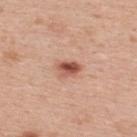Captured during whole-body skin photography for melanoma surveillance; the lesion was not biopsied.
From the upper back.
The subject is a female approximately 30 years of age.
The recorded lesion diameter is about 2.5 mm.
A region of skin cropped from a whole-body photographic capture, roughly 15 mm wide.